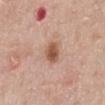– location — the abdomen
– subject — male, aged approximately 60
– lighting — white-light illumination
– image source — ~15 mm crop, total-body skin-cancer survey